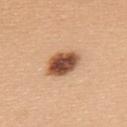This lesion was catalogued during total-body skin photography and was not selected for biopsy. The subject is a female approximately 45 years of age. This image is a 15 mm lesion crop taken from a total-body photograph. About 4 mm across. The tile uses white-light illumination. Located on the upper back.